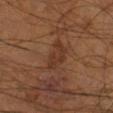workup: catalogued during a skin exam; not biopsied
image: total-body-photography crop, ~15 mm field of view
lesion size: ~4 mm (longest diameter)
illumination: cross-polarized illumination
automated lesion analysis: an area of roughly 8.5 mm², a shape eccentricity near 0.8, and a shape-asymmetry score of about 0.25 (0 = symmetric); an average lesion color of about L≈32 a*≈18 b*≈26 (CIELAB), a lesion–skin lightness drop of about 6, and a normalized lesion–skin contrast near 6
location: the right lower leg
subject: male, in their mid-50s